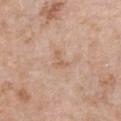The lesion was tiled from a total-body skin photograph and was not biopsied.
The lesion is located on the chest.
The patient is a female in their 40s.
This image is a 15 mm lesion crop taken from a total-body photograph.